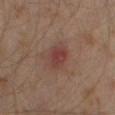notes = catalogued during a skin exam; not biopsied | patient = male, in their 60s | lesion size = ~3 mm (longest diameter) | image-analysis metrics = a footprint of about 5.5 mm², a shape eccentricity near 0.65, and a symmetry-axis asymmetry near 0.2; a lesion color around L≈35 a*≈21 b*≈21 in CIELAB, about 7 CIELAB-L* units darker than the surrounding skin, and a normalized border contrast of about 7; border irregularity of about 1.5 on a 0–10 scale and peripheral color asymmetry of about 0.5 | body site = the left thigh | imaging modality = total-body-photography crop, ~15 mm field of view.